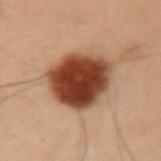Impression: The lesion was photographed on a routine skin check and not biopsied; there is no pathology result. Clinical summary: The subject is a male in their mid-50s. This image is a 15 mm lesion crop taken from a total-body photograph. The lesion's longest dimension is about 6.5 mm. This is a cross-polarized tile. An algorithmic analysis of the crop reported a shape eccentricity near 0.65 and a shape-asymmetry score of about 0.15 (0 = symmetric). And it measured a classifier nevus-likeness of about 100/100 and a detector confidence of about 100 out of 100 that the crop contains a lesion. Located on the left upper arm.